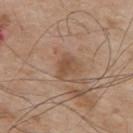Findings:
- lesion size · about 3 mm
- subject · male, about 75 years old
- body site · the mid back
- image source · 15 mm crop, total-body photography
- automated lesion analysis · a lesion area of about 4.5 mm² and a symmetry-axis asymmetry near 0.35; an average lesion color of about L≈50 a*≈18 b*≈30 (CIELAB) and a lesion-to-skin contrast of about 6.5 (normalized; higher = more distinct); a classifier nevus-likeness of about 10/100 and lesion-presence confidence of about 100/100